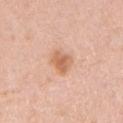Case summary:
- biopsy status — catalogued during a skin exam; not biopsied
- TBP lesion metrics — an area of roughly 6 mm², an eccentricity of roughly 0.7, and a shape-asymmetry score of about 0.25 (0 = symmetric); a classifier nevus-likeness of about 80/100
- imaging modality — total-body-photography crop, ~15 mm field of view
- subject — female, roughly 35 years of age
- location — the right upper arm
- lesion diameter — ~3.5 mm (longest diameter)
- illumination — white-light